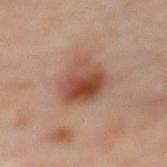notes: total-body-photography surveillance lesion; no biopsy
illumination: cross-polarized
location: the mid back
subject: female, in their 60s
acquisition: ~15 mm tile from a whole-body skin photo
size: ≈5 mm